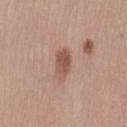This lesion was catalogued during total-body skin photography and was not selected for biopsy.
Located on the left thigh.
This image is a 15 mm lesion crop taken from a total-body photograph.
A female patient about 35 years old.
Approximately 3.5 mm at its widest.
Automated image analysis of the tile measured a footprint of about 5.5 mm², an outline eccentricity of about 0.85 (0 = round, 1 = elongated), and two-axis asymmetry of about 0.2.
This is a white-light tile.